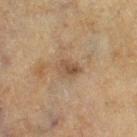Q: Was this lesion biopsied?
A: no biopsy performed (imaged during a skin exam)
Q: What is the imaging modality?
A: ~15 mm crop, total-body skin-cancer survey
Q: Lesion size?
A: ≈2.5 mm
Q: Patient demographics?
A: female, in their mid- to late 50s
Q: Lesion location?
A: the leg